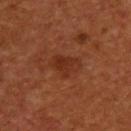Recorded during total-body skin imaging; not selected for excision or biopsy. Automated tile analysis of the lesion measured an eccentricity of roughly 0.65. The analysis additionally found an average lesion color of about L≈29 a*≈24 b*≈29 (CIELAB) and a normalized lesion–skin contrast near 6. And it measured border irregularity of about 3.5 on a 0–10 scale, internal color variation of about 3 on a 0–10 scale, and radial color variation of about 1. The software also gave a nevus-likeness score of about 5/100 and lesion-presence confidence of about 100/100. On the upper back. About 4 mm across. Cropped from a whole-body photographic skin survey; the tile spans about 15 mm. A male subject, about 55 years old. Imaged with cross-polarized lighting.